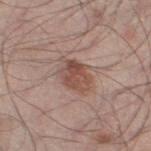Notes:
- notes: imaged on a skin check; not biopsied
- lesion size: ~4 mm (longest diameter)
- acquisition: total-body-photography crop, ~15 mm field of view
- subject: male, in their mid-50s
- tile lighting: white-light illumination
- site: the leg